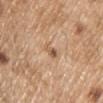* notes · no biopsy performed (imaged during a skin exam)
* subject · male, aged approximately 70
* image-analysis metrics · border irregularity of about 3 on a 0–10 scale, a color-variation rating of about 8/10, and peripheral color asymmetry of about 3; a classifier nevus-likeness of about 5/100 and lesion-presence confidence of about 100/100
* image source · total-body-photography crop, ~15 mm field of view
* site · the front of the torso
* lighting · white-light illumination